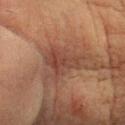<lesion>
<biopsy_status>not biopsied; imaged during a skin examination</biopsy_status>
<site>head or neck</site>
<lighting>cross-polarized</lighting>
<patient>
  <sex>female</sex>
  <age_approx>80</age_approx>
</patient>
<lesion_size>
  <long_diameter_mm_approx>5.0</long_diameter_mm_approx>
</lesion_size>
<image>
  <source>total-body photography crop</source>
  <field_of_view_mm>15</field_of_view_mm>
</image>
</lesion>